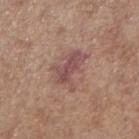{
  "biopsy_status": "not biopsied; imaged during a skin examination",
  "automated_metrics": {
    "area_mm2_approx": 9.5,
    "shape_asymmetry": 0.35,
    "nevus_likeness_0_100": 5
  },
  "image": {
    "source": "total-body photography crop",
    "field_of_view_mm": 15
  },
  "patient": {
    "sex": "female",
    "age_approx": 65
  },
  "lesion_size": {
    "long_diameter_mm_approx": 4.5
  },
  "site": "right forearm",
  "lighting": "white-light"
}